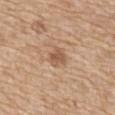  biopsy_status: not biopsied; imaged during a skin examination
  image:
    source: total-body photography crop
    field_of_view_mm: 15
  patient:
    sex: male
    age_approx: 70
  site: front of the torso
  lighting: white-light